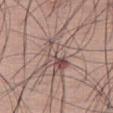Recorded during total-body skin imaging; not selected for excision or biopsy. The lesion is on the right thigh. A male patient approximately 60 years of age. A close-up tile cropped from a whole-body skin photograph, about 15 mm across. Longest diameter approximately 6.5 mm. The tile uses white-light illumination.